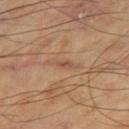  biopsy_status: not biopsied; imaged during a skin examination
  lesion_size:
    long_diameter_mm_approx: 2.5
  automated_metrics:
    area_mm2_approx: 2.0
    eccentricity: 0.9
    cielab_L: 52
    cielab_a: 21
    cielab_b: 31
    vs_skin_darker_L: 7.0
    color_variation_0_10: 0.0
    peripheral_color_asymmetry: 0.0
  site: leg
  lighting: cross-polarized
  image:
    source: total-body photography crop
    field_of_view_mm: 15
  patient:
    sex: male
    age_approx: 65